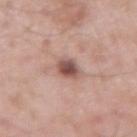| field | value |
|---|---|
| biopsy status | imaged on a skin check; not biopsied |
| image source | total-body-photography crop, ~15 mm field of view |
| location | the right upper arm |
| size | ≈3.5 mm |
| lighting | white-light illumination |
| patient | male, about 55 years old |
| TBP lesion metrics | a shape eccentricity near 0.75 and a symmetry-axis asymmetry near 0.25; roughly 14 lightness units darker than nearby skin and a normalized lesion–skin contrast near 9.5; a classifier nevus-likeness of about 90/100 and a detector confidence of about 100 out of 100 that the crop contains a lesion |